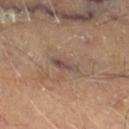Q: Was a biopsy performed?
A: catalogued during a skin exam; not biopsied
Q: Lesion location?
A: the right thigh
Q: Automated lesion metrics?
A: roughly 8 lightness units darker than nearby skin and a normalized lesion–skin contrast near 8; border irregularity of about 3 on a 0–10 scale, internal color variation of about 2 on a 0–10 scale, and peripheral color asymmetry of about 0.5
Q: What is the imaging modality?
A: ~15 mm tile from a whole-body skin photo
Q: Who is the patient?
A: male, aged 68–72